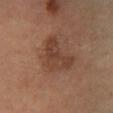Q: Was this lesion biopsied?
A: no biopsy performed (imaged during a skin exam)
Q: Illumination type?
A: cross-polarized illumination
Q: How was this image acquired?
A: total-body-photography crop, ~15 mm field of view
Q: What did automated image analysis measure?
A: a shape eccentricity near 0.8 and two-axis asymmetry of about 0.5; a classifier nevus-likeness of about 5/100
Q: Where on the body is the lesion?
A: the leg
Q: What is the lesion's diameter?
A: about 5.5 mm
Q: Who is the patient?
A: male, approximately 55 years of age